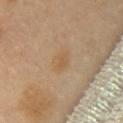Q: Was this lesion biopsied?
A: no biopsy performed (imaged during a skin exam)